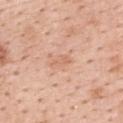Case summary:
• notes · imaged on a skin check; not biopsied
• automated metrics · a detector confidence of about 100 out of 100 that the crop contains a lesion
• lighting · white-light illumination
• patient · female, aged approximately 45
• body site · the upper back
• image source · ~15 mm crop, total-body skin-cancer survey
• lesion size · ≈2.5 mm